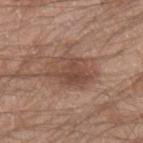Findings:
• workup · total-body-photography surveillance lesion; no biopsy
• image · total-body-photography crop, ~15 mm field of view
• body site · the left forearm
• tile lighting · white-light
• patient · male, roughly 20 years of age
• automated metrics · a lesion area of about 16 mm² and an eccentricity of roughly 0.75; about 9 CIELAB-L* units darker than the surrounding skin; a border-irregularity index near 2.5/10 and a peripheral color-asymmetry measure near 1.5; an automated nevus-likeness rating near 5 out of 100 and a detector confidence of about 100 out of 100 that the crop contains a lesion
• lesion diameter · about 6 mm